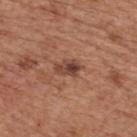notes = no biopsy performed (imaged during a skin exam) | subject = male, aged around 65 | imaging modality = ~15 mm crop, total-body skin-cancer survey | location = the upper back | size = ≈3 mm.